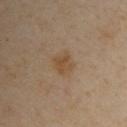| key | value |
|---|---|
| workup | total-body-photography surveillance lesion; no biopsy |
| lighting | cross-polarized illumination |
| location | the left upper arm |
| patient | male, approximately 50 years of age |
| image | ~15 mm crop, total-body skin-cancer survey |
| size | about 2.5 mm |